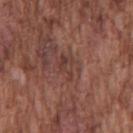notes: imaged on a skin check; not biopsied | anatomic site: the chest | automated metrics: an area of roughly 3.5 mm² and an eccentricity of roughly 0.8; a border-irregularity rating of about 4.5/10, a color-variation rating of about 3.5/10, and a peripheral color-asymmetry measure near 1 | tile lighting: white-light | image: total-body-photography crop, ~15 mm field of view | subject: male, in their mid- to late 70s | lesion diameter: about 3 mm.